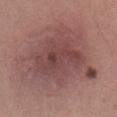{
  "biopsy_status": "not biopsied; imaged during a skin examination",
  "image": {
    "source": "total-body photography crop",
    "field_of_view_mm": 15
  },
  "patient": {
    "sex": "female",
    "age_approx": 30
  },
  "site": "lower back",
  "lighting": "white-light",
  "lesion_size": {
    "long_diameter_mm_approx": 10.5
  }
}